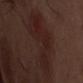Assessment: No biopsy was performed on this lesion — it was imaged during a full skin examination and was not determined to be concerning. Clinical summary: A 15 mm crop from a total-body photograph taken for skin-cancer surveillance. A male patient aged 68 to 72. The lesion is on the abdomen. The recorded lesion diameter is about 8 mm. The tile uses white-light illumination.